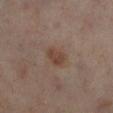Captured during whole-body skin photography for melanoma surveillance; the lesion was not biopsied.
About 2.5 mm across.
The lesion is on the left lower leg.
The tile uses cross-polarized illumination.
Cropped from a total-body skin-imaging series; the visible field is about 15 mm.
The subject is a female roughly 50 years of age.